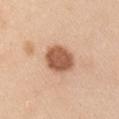| feature | finding |
|---|---|
| biopsy status | no biopsy performed (imaged during a skin exam) |
| tile lighting | white-light |
| body site | the left upper arm |
| imaging modality | ~15 mm tile from a whole-body skin photo |
| size | ~4 mm (longest diameter) |
| patient | male, aged approximately 35 |